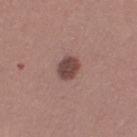Assessment: Captured during whole-body skin photography for melanoma surveillance; the lesion was not biopsied. Acquisition and patient details: A female subject aged around 35. The lesion's longest dimension is about 3 mm. Located on the leg. Cropped from a total-body skin-imaging series; the visible field is about 15 mm. Captured under white-light illumination.